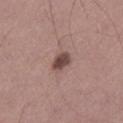• workup: total-body-photography surveillance lesion; no biopsy
• imaging modality: total-body-photography crop, ~15 mm field of view
• subject: male, aged approximately 45
• body site: the left lower leg